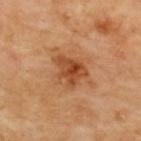• workup: no biopsy performed (imaged during a skin exam)
• anatomic site: the upper back
• acquisition: ~15 mm tile from a whole-body skin photo
• lighting: cross-polarized illumination
• diameter: ~4 mm (longest diameter)